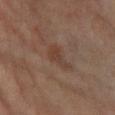{"biopsy_status": "not biopsied; imaged during a skin examination", "image": {"source": "total-body photography crop", "field_of_view_mm": 15}, "lesion_size": {"long_diameter_mm_approx": 4.0}, "lighting": "cross-polarized", "automated_metrics": {"eccentricity": 0.9, "shape_asymmetry": 0.55, "nevus_likeness_0_100": 0, "lesion_detection_confidence_0_100": 100}, "patient": {"sex": "female", "age_approx": 65}, "site": "left leg"}